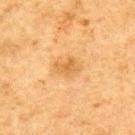The total-body-photography lesion software estimated an outline eccentricity of about 0.7 (0 = round, 1 = elongated) and a shape-asymmetry score of about 0.25 (0 = symmetric). And it measured border irregularity of about 3 on a 0–10 scale, a within-lesion color-variation index near 2/10, and peripheral color asymmetry of about 0.5.
A male patient, aged approximately 75.
The lesion is located on the upper back.
Imaged with cross-polarized lighting.
Cropped from a total-body skin-imaging series; the visible field is about 15 mm.